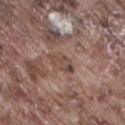Captured during whole-body skin photography for melanoma surveillance; the lesion was not biopsied. Approximately 3.5 mm at its widest. This image is a 15 mm lesion crop taken from a total-body photograph. On the leg. An algorithmic analysis of the crop reported a lesion area of about 5 mm², a shape eccentricity near 0.8, and two-axis asymmetry of about 0.3. The software also gave a lesion color around L≈46 a*≈17 b*≈23 in CIELAB and a lesion–skin lightness drop of about 8. The analysis additionally found a classifier nevus-likeness of about 0/100. Imaged with white-light lighting. A male patient roughly 75 years of age.